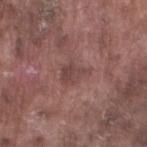Longest diameter approximately 3.5 mm.
A male subject roughly 75 years of age.
A region of skin cropped from a whole-body photographic capture, roughly 15 mm wide.
The lesion is on the left lower leg.
The lesion-visualizer software estimated a lesion area of about 5.5 mm², an eccentricity of roughly 0.75, and two-axis asymmetry of about 0.4. The software also gave an automated nevus-likeness rating near 0 out of 100 and a lesion-detection confidence of about 95/100.